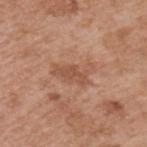Q: Was a biopsy performed?
A: imaged on a skin check; not biopsied
Q: What are the patient's age and sex?
A: male, aged 53 to 57
Q: What is the anatomic site?
A: the upper back
Q: What kind of image is this?
A: total-body-photography crop, ~15 mm field of view